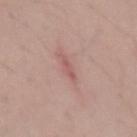The lesion was photographed on a routine skin check and not biopsied; there is no pathology result. Imaged with white-light lighting. This image is a 15 mm lesion crop taken from a total-body photograph. The lesion-visualizer software estimated a footprint of about 3 mm² and a symmetry-axis asymmetry near 0.35. The analysis additionally found a classifier nevus-likeness of about 0/100. A male subject, in their mid-20s. The lesion is on the left forearm. The recorded lesion diameter is about 3 mm.